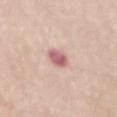Impression:
No biopsy was performed on this lesion — it was imaged during a full skin examination and was not determined to be concerning.
Clinical summary:
An algorithmic analysis of the crop reported a mean CIELAB color near L≈61 a*≈26 b*≈20 and a normalized border contrast of about 9. It also reported a border-irregularity index near 2/10 and radial color variation of about 1.5. Approximately 3 mm at its widest. A female patient aged 63–67. Cropped from a whole-body photographic skin survey; the tile spans about 15 mm. Captured under white-light illumination. On the lower back.